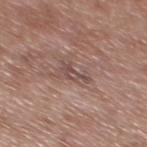Notes:
– imaging modality · ~15 mm tile from a whole-body skin photo
– lighting · white-light illumination
– anatomic site · the mid back
– patient · male, aged 48–52